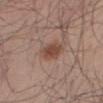Imaged during a routine full-body skin examination; the lesion was not biopsied and no histopathology is available.
The lesion's longest dimension is about 3.5 mm.
Imaged with white-light lighting.
A male subject, in their mid- to late 40s.
On the leg.
A 15 mm close-up tile from a total-body photography series done for melanoma screening.